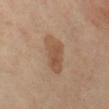{"patient": {"sex": "female", "age_approx": 50}, "lighting": "cross-polarized", "lesion_size": {"long_diameter_mm_approx": 4.0}, "image": {"source": "total-body photography crop", "field_of_view_mm": 15}, "automated_metrics": {"area_mm2_approx": 10.0, "border_irregularity_0_10": 3.5, "color_variation_0_10": 3.0, "peripheral_color_asymmetry": 1.0, "nevus_likeness_0_100": 80, "lesion_detection_confidence_0_100": 100}, "site": "left thigh"}